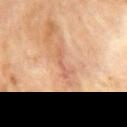Impression:
Captured during whole-body skin photography for melanoma surveillance; the lesion was not biopsied.
Clinical summary:
The recorded lesion diameter is about 3.5 mm. The subject is a male roughly 70 years of age. This image is a 15 mm lesion crop taken from a total-body photograph. The lesion is located on the back. The total-body-photography lesion software estimated an area of roughly 3.5 mm², an outline eccentricity of about 0.95 (0 = round, 1 = elongated), and two-axis asymmetry of about 0.45. The software also gave an average lesion color of about L≈61 a*≈24 b*≈34 (CIELAB), roughly 8 lightness units darker than nearby skin, and a normalized lesion–skin contrast near 5. It also reported a detector confidence of about 80 out of 100 that the crop contains a lesion.